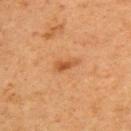Assessment:
The lesion was photographed on a routine skin check and not biopsied; there is no pathology result.
Background:
A close-up tile cropped from a whole-body skin photograph, about 15 mm across. From the upper back. The subject is a female in their 60s. Measured at roughly 3 mm in maximum diameter.